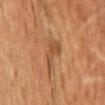{"biopsy_status": "not biopsied; imaged during a skin examination", "lesion_size": {"long_diameter_mm_approx": 4.5}, "site": "chest", "lighting": "cross-polarized", "patient": {"sex": "male", "age_approx": 60}, "automated_metrics": {"area_mm2_approx": 6.0, "shape_asymmetry": 0.5, "border_irregularity_0_10": 5.5, "color_variation_0_10": 1.5, "peripheral_color_asymmetry": 0.5, "lesion_detection_confidence_0_100": 90}, "image": {"source": "total-body photography crop", "field_of_view_mm": 15}}